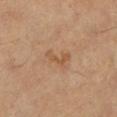The lesion was photographed on a routine skin check and not biopsied; there is no pathology result. A male subject roughly 60 years of age. This is a cross-polarized tile. Automated tile analysis of the lesion measured a footprint of about 3.5 mm² and an eccentricity of roughly 0.95. The software also gave a lesion-to-skin contrast of about 6 (normalized; higher = more distinct). The software also gave a within-lesion color-variation index near 0/10 and a peripheral color-asymmetry measure near 0. A 15 mm crop from a total-body photograph taken for skin-cancer surveillance. The lesion is located on the right lower leg. Measured at roughly 3.5 mm in maximum diameter.